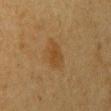- biopsy status — total-body-photography surveillance lesion; no biopsy
- image-analysis metrics — an eccentricity of roughly 0.6 and two-axis asymmetry of about 0.25; border irregularity of about 2.5 on a 0–10 scale, a within-lesion color-variation index near 2.5/10, and peripheral color asymmetry of about 1
- anatomic site — the arm
- size — about 3 mm
- patient — female, aged 53 to 57
- acquisition — ~15 mm tile from a whole-body skin photo